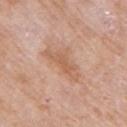imaging modality: total-body-photography crop, ~15 mm field of view
subject: female, aged 68 to 72
anatomic site: the right upper arm
tile lighting: white-light illumination
automated metrics: about 8 CIELAB-L* units darker than the surrounding skin and a normalized lesion–skin contrast near 6; a classifier nevus-likeness of about 0/100 and a lesion-detection confidence of about 100/100
lesion diameter: ≈5 mm